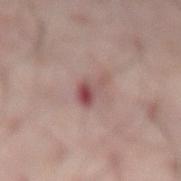Q: Is there a histopathology result?
A: imaged on a skin check; not biopsied
Q: Where on the body is the lesion?
A: the abdomen
Q: Illumination type?
A: cross-polarized illumination
Q: Patient demographics?
A: male, in their 50s
Q: What kind of image is this?
A: total-body-photography crop, ~15 mm field of view
Q: How large is the lesion?
A: ≈4 mm